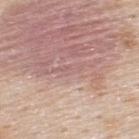workup: imaged on a skin check; not biopsied
subject: male, aged around 45
tile lighting: white-light illumination
size: ≈4 mm
acquisition: ~15 mm crop, total-body skin-cancer survey
body site: the upper back